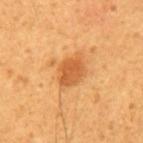Recorded during total-body skin imaging; not selected for excision or biopsy.
Imaged with cross-polarized lighting.
A male patient aged 43–47.
From the mid back.
Measured at roughly 4 mm in maximum diameter.
This image is a 15 mm lesion crop taken from a total-body photograph.
The total-body-photography lesion software estimated an area of roughly 8 mm² and a shape eccentricity near 0.7. The analysis additionally found a border-irregularity index near 2/10, internal color variation of about 2.5 on a 0–10 scale, and peripheral color asymmetry of about 1. It also reported an automated nevus-likeness rating near 90 out of 100 and a lesion-detection confidence of about 100/100.